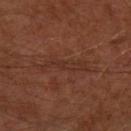Measured at roughly 5.5 mm in maximum diameter.
The lesion-visualizer software estimated a lesion area of about 6 mm², a shape eccentricity near 0.95, and a shape-asymmetry score of about 0.45 (0 = symmetric). And it measured border irregularity of about 7 on a 0–10 scale, a color-variation rating of about 0.5/10, and radial color variation of about 0. The analysis additionally found a classifier nevus-likeness of about 0/100.
Cropped from a total-body skin-imaging series; the visible field is about 15 mm.
A male subject about 60 years old.
From the left forearm.